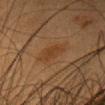Recorded during total-body skin imaging; not selected for excision or biopsy.